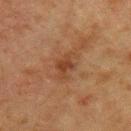notes — catalogued during a skin exam; not biopsied
lesion size — about 3 mm
illumination — cross-polarized illumination
image source — ~15 mm tile from a whole-body skin photo
patient — female, approximately 55 years of age
automated lesion analysis — a lesion area of about 5 mm² and a shape eccentricity near 0.8; a mean CIELAB color near L≈34 a*≈19 b*≈28, a lesion–skin lightness drop of about 7, and a lesion-to-skin contrast of about 6.5 (normalized; higher = more distinct); border irregularity of about 4 on a 0–10 scale; a classifier nevus-likeness of about 10/100 and a detector confidence of about 100 out of 100 that the crop contains a lesion
body site — the upper back